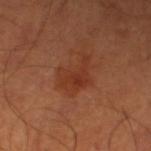Part of a total-body skin-imaging series; this lesion was reviewed on a skin check and was not flagged for biopsy. The subject is a male aged around 50. A roughly 15 mm field-of-view crop from a total-body skin photograph. Automated image analysis of the tile measured a footprint of about 8 mm², a shape eccentricity near 0.75, and a symmetry-axis asymmetry near 0.6. It also reported a border-irregularity rating of about 7/10, internal color variation of about 3 on a 0–10 scale, and peripheral color asymmetry of about 1. The analysis additionally found a detector confidence of about 100 out of 100 that the crop contains a lesion. The lesion's longest dimension is about 4.5 mm. On the left lower leg.